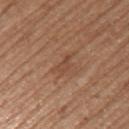Findings:
• biopsy status: imaged on a skin check; not biopsied
• subject: female, aged around 75
• anatomic site: the left upper arm
• acquisition: ~15 mm tile from a whole-body skin photo